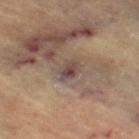{"biopsy_status": "not biopsied; imaged during a skin examination", "patient": {"sex": "female", "age_approx": 65}, "site": "left leg", "automated_metrics": {"cielab_L": 44, "cielab_a": 14, "cielab_b": 20, "vs_skin_contrast_norm": 8.0}, "image": {"source": "total-body photography crop", "field_of_view_mm": 15}, "lesion_size": {"long_diameter_mm_approx": 3.0}}